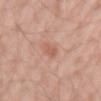Cropped from a whole-body photographic skin survey; the tile spans about 15 mm. The lesion-visualizer software estimated an area of roughly 2.5 mm², an eccentricity of roughly 0.85, and a shape-asymmetry score of about 0.2 (0 = symmetric). Approximately 2.5 mm at its widest. A male subject roughly 60 years of age. The lesion is located on the arm. Imaged with white-light lighting.